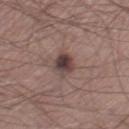notes — catalogued during a skin exam; not biopsied
image source — total-body-photography crop, ~15 mm field of view
image-analysis metrics — a footprint of about 5.5 mm², a shape eccentricity near 0.75, and a symmetry-axis asymmetry near 0.2; a border-irregularity index near 2.5/10 and internal color variation of about 5 on a 0–10 scale
diameter — ≈3.5 mm
body site — the left thigh
illumination — white-light
subject — male, aged 53 to 57